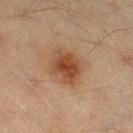<tbp_lesion>
<biopsy_status>not biopsied; imaged during a skin examination</biopsy_status>
<image>
  <source>total-body photography crop</source>
  <field_of_view_mm>15</field_of_view_mm>
</image>
<lighting>cross-polarized</lighting>
<patient>
  <sex>male</sex>
  <age_approx>40</age_approx>
</patient>
<site>right thigh</site>
</tbp_lesion>